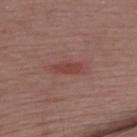Findings:
– notes · total-body-photography surveillance lesion; no biopsy
– image · ~15 mm crop, total-body skin-cancer survey
– diameter · ~4.5 mm (longest diameter)
– subject · male, about 55 years old
– location · the upper back